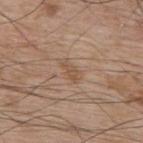Imaged during a routine full-body skin examination; the lesion was not biopsied and no histopathology is available. A 15 mm crop from a total-body photograph taken for skin-cancer surveillance. A male patient aged around 70. An algorithmic analysis of the crop reported an area of roughly 2.5 mm², a shape eccentricity near 0.9, and a shape-asymmetry score of about 0.45 (0 = symmetric). The analysis additionally found a border-irregularity index near 5/10 and a color-variation rating of about 0/10. The tile uses white-light illumination. The lesion's longest dimension is about 2.5 mm. The lesion is located on the back.